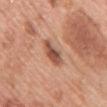Imaged during a routine full-body skin examination; the lesion was not biopsied and no histopathology is available. A 15 mm close-up tile from a total-body photography series done for melanoma screening. The tile uses white-light illumination. The subject is a female roughly 60 years of age. The recorded lesion diameter is about 4 mm. On the chest.